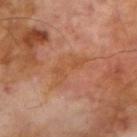Assessment:
The lesion was photographed on a routine skin check and not biopsied; there is no pathology result.
Background:
A 15 mm close-up extracted from a 3D total-body photography capture. The lesion is located on the right upper arm. A male subject, roughly 70 years of age.